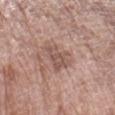{"biopsy_status": "not biopsied; imaged during a skin examination", "patient": {"sex": "female", "age_approx": 70}, "lesion_size": {"long_diameter_mm_approx": 4.5}, "site": "left upper arm", "lighting": "white-light", "image": {"source": "total-body photography crop", "field_of_view_mm": 15}, "automated_metrics": {"area_mm2_approx": 8.5, "eccentricity": 0.8, "shape_asymmetry": 0.35, "cielab_L": 54, "cielab_a": 19, "cielab_b": 24, "vs_skin_darker_L": 9.0, "border_irregularity_0_10": 4.5, "color_variation_0_10": 3.0, "peripheral_color_asymmetry": 1.0, "nevus_likeness_0_100": 0, "lesion_detection_confidence_0_100": 100}}